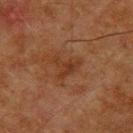follow-up: imaged on a skin check; not biopsied
subject: male, aged 63 to 67
tile lighting: cross-polarized
anatomic site: the back
image-analysis metrics: an area of roughly 6.5 mm², a shape eccentricity near 0.6, and a symmetry-axis asymmetry near 0.55; a lesion color around L≈29 a*≈19 b*≈27 in CIELAB, about 5 CIELAB-L* units darker than the surrounding skin, and a normalized border contrast of about 6
acquisition: total-body-photography crop, ~15 mm field of view
lesion size: about 3.5 mm